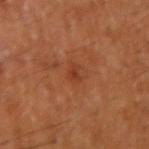Q: Where on the body is the lesion?
A: the arm
Q: What are the patient's age and sex?
A: male, aged 58–62
Q: What is the imaging modality?
A: ~15 mm tile from a whole-body skin photo
Q: Lesion size?
A: ~1.5 mm (longest diameter)